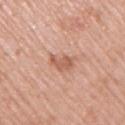Impression:
Imaged during a routine full-body skin examination; the lesion was not biopsied and no histopathology is available.
Acquisition and patient details:
A roughly 15 mm field-of-view crop from a total-body skin photograph. The total-body-photography lesion software estimated a mean CIELAB color near L≈59 a*≈24 b*≈31 and a lesion-to-skin contrast of about 6.5 (normalized; higher = more distinct). The analysis additionally found a nevus-likeness score of about 0/100 and a detector confidence of about 100 out of 100 that the crop contains a lesion. The recorded lesion diameter is about 3.5 mm. Imaged with white-light lighting. A male subject, roughly 55 years of age. Located on the right upper arm.